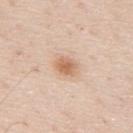follow-up: imaged on a skin check; not biopsied
lesion diameter: about 2.5 mm
location: the mid back
lighting: white-light illumination
image source: ~15 mm crop, total-body skin-cancer survey
subject: male, about 80 years old